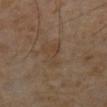Impression: The lesion was tiled from a total-body skin photograph and was not biopsied. Image and clinical context: The patient is a male aged around 65. The lesion-visualizer software estimated a lesion area of about 5 mm², a shape eccentricity near 0.9, and a shape-asymmetry score of about 0.5 (0 = symmetric). The analysis additionally found border irregularity of about 5.5 on a 0–10 scale, a color-variation rating of about 1.5/10, and a peripheral color-asymmetry measure near 0.5. The software also gave an automated nevus-likeness rating near 0 out of 100 and a detector confidence of about 100 out of 100 that the crop contains a lesion. The tile uses cross-polarized illumination. Measured at roughly 4 mm in maximum diameter. A 15 mm close-up tile from a total-body photography series done for melanoma screening.